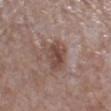The lesion was tiled from a total-body skin photograph and was not biopsied.
A female patient aged 68–72.
A roughly 15 mm field-of-view crop from a total-body skin photograph.
From the right lower leg.